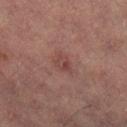Assessment: This lesion was catalogued during total-body skin photography and was not selected for biopsy. Image and clinical context: The lesion is located on the right lower leg. Automated tile analysis of the lesion measured a lesion color around L≈35 a*≈19 b*≈18 in CIELAB, roughly 6 lightness units darker than nearby skin, and a lesion-to-skin contrast of about 5.5 (normalized; higher = more distinct). It also reported a nevus-likeness score of about 0/100 and lesion-presence confidence of about 100/100. The recorded lesion diameter is about 3 mm. The subject is a female aged 68–72. A roughly 15 mm field-of-view crop from a total-body skin photograph. Captured under cross-polarized illumination.